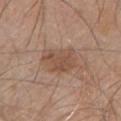Case summary:
• biopsy status · total-body-photography surveillance lesion; no biopsy
• anatomic site · the chest
• size · ~4.5 mm (longest diameter)
• automated lesion analysis · an average lesion color of about L≈51 a*≈18 b*≈29 (CIELAB), roughly 8 lightness units darker than nearby skin, and a lesion-to-skin contrast of about 6 (normalized; higher = more distinct); a classifier nevus-likeness of about 15/100 and lesion-presence confidence of about 100/100
• patient · male, about 80 years old
• imaging modality · ~15 mm crop, total-body skin-cancer survey
• lighting · white-light illumination